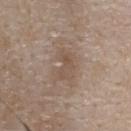| key | value |
|---|---|
| biopsy status | no biopsy performed (imaged during a skin exam) |
| acquisition | total-body-photography crop, ~15 mm field of view |
| subject | female, about 55 years old |
| automated lesion analysis | an average lesion color of about L≈51 a*≈14 b*≈26 (CIELAB), about 7 CIELAB-L* units darker than the surrounding skin, and a normalized border contrast of about 5.5; border irregularity of about 4 on a 0–10 scale, internal color variation of about 3 on a 0–10 scale, and peripheral color asymmetry of about 1 |
| body site | the back |
| tile lighting | white-light |